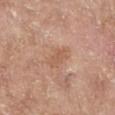Assessment: Recorded during total-body skin imaging; not selected for excision or biopsy. Context: The lesion's longest dimension is about 3.5 mm. A 15 mm close-up extracted from a 3D total-body photography capture. The subject is a female aged 73–77. The lesion is on the left lower leg. This is a white-light tile. An algorithmic analysis of the crop reported a normalized border contrast of about 5.5.